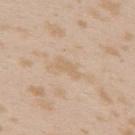Impression:
Recorded during total-body skin imaging; not selected for excision or biopsy.
Context:
The lesion is on the upper back. A female subject aged 23–27. This is a white-light tile. The recorded lesion diameter is about 3 mm. A 15 mm crop from a total-body photograph taken for skin-cancer surveillance.